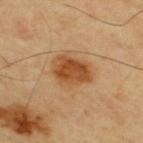Part of a total-body skin-imaging series; this lesion was reviewed on a skin check and was not flagged for biopsy.